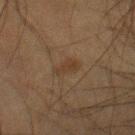The lesion was photographed on a routine skin check and not biopsied; there is no pathology result. A lesion tile, about 15 mm wide, cut from a 3D total-body photograph. An algorithmic analysis of the crop reported a border-irregularity rating of about 3/10, a within-lesion color-variation index near 1/10, and radial color variation of about 0.5. The software also gave an automated nevus-likeness rating near 10 out of 100. About 3 mm across. From the right lower leg. Imaged with cross-polarized lighting. The subject is a male aged 33 to 37.